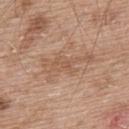site — the back
automated lesion analysis — an average lesion color of about L≈56 a*≈19 b*≈31 (CIELAB), roughly 7 lightness units darker than nearby skin, and a normalized lesion–skin contrast near 5.5; internal color variation of about 2 on a 0–10 scale and a peripheral color-asymmetry measure near 0.5; a nevus-likeness score of about 0/100 and a detector confidence of about 85 out of 100 that the crop contains a lesion
tile lighting — white-light
patient — male, in their mid- to late 60s
image — ~15 mm crop, total-body skin-cancer survey
lesion size — ≈6 mm